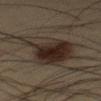Q: Is there a histopathology result?
A: no biopsy performed (imaged during a skin exam)
Q: What are the patient's age and sex?
A: male, approximately 35 years of age
Q: What is the imaging modality?
A: 15 mm crop, total-body photography
Q: Lesion size?
A: ≈7 mm
Q: What did automated image analysis measure?
A: a mean CIELAB color near L≈24 a*≈10 b*≈18 and a lesion–skin lightness drop of about 9; a within-lesion color-variation index near 8/10 and peripheral color asymmetry of about 3
Q: Lesion location?
A: the mid back